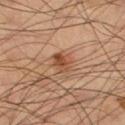The lesion was photographed on a routine skin check and not biopsied; there is no pathology result. Imaged with cross-polarized lighting. A male subject, aged 58 to 62. A 15 mm close-up tile from a total-body photography series done for melanoma screening. On the left thigh.